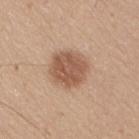The lesion was tiled from a total-body skin photograph and was not biopsied. A male subject aged around 45. The tile uses white-light illumination. Approximately 4.5 mm at its widest. On the right upper arm. Cropped from a whole-body photographic skin survey; the tile spans about 15 mm.